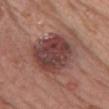– workup — imaged on a skin check; not biopsied
– body site — the front of the torso
– lesion size — about 7 mm
– automated lesion analysis — about 12 CIELAB-L* units darker than the surrounding skin and a lesion-to-skin contrast of about 9.5 (normalized; higher = more distinct); a within-lesion color-variation index near 7.5/10 and a peripheral color-asymmetry measure near 2.5; an automated nevus-likeness rating near 50 out of 100
– image — 15 mm crop, total-body photography
– illumination — white-light illumination
– patient — female, in their 60s